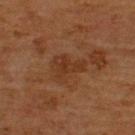Imaged during a routine full-body skin examination; the lesion was not biopsied and no histopathology is available. A male patient approximately 65 years of age. On the upper back. This is a cross-polarized tile. A roughly 15 mm field-of-view crop from a total-body skin photograph. Approximately 4 mm at its widest.